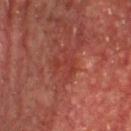The lesion was tiled from a total-body skin photograph and was not biopsied.